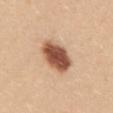workup = catalogued during a skin exam; not biopsied | lighting = white-light illumination | lesion diameter = ≈5 mm | body site = the mid back | image = ~15 mm crop, total-body skin-cancer survey | subject = female, aged 23 to 27.